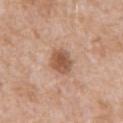Recorded during total-body skin imaging; not selected for excision or biopsy. A 15 mm close-up tile from a total-body photography series done for melanoma screening. About 3 mm across. From the mid back. The total-body-photography lesion software estimated an outline eccentricity of about 0.6 (0 = round, 1 = elongated) and two-axis asymmetry of about 0.2. The software also gave a nevus-likeness score of about 85/100 and a detector confidence of about 100 out of 100 that the crop contains a lesion. A male patient about 60 years old. Captured under white-light illumination.